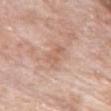subject — female, about 75 years old | location — the front of the torso | illumination — white-light illumination | lesion size — ≈3 mm | automated lesion analysis — an average lesion color of about L≈61 a*≈20 b*≈30 (CIELAB), a lesion–skin lightness drop of about 7, and a lesion-to-skin contrast of about 5 (normalized; higher = more distinct); a border-irregularity rating of about 3/10 and a within-lesion color-variation index near 1.5/10; a nevus-likeness score of about 0/100 and a detector confidence of about 100 out of 100 that the crop contains a lesion | image source — 15 mm crop, total-body photography.